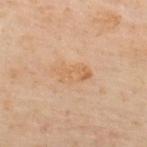Findings:
– workup — no biopsy performed (imaged during a skin exam)
– diameter — ≈4 mm
– anatomic site — the upper back
– patient — male, aged 48 to 52
– tile lighting — cross-polarized
– imaging modality — total-body-photography crop, ~15 mm field of view
– automated metrics — a footprint of about 6.5 mm², an eccentricity of roughly 0.85, and a shape-asymmetry score of about 0.3 (0 = symmetric); an automated nevus-likeness rating near 0 out of 100